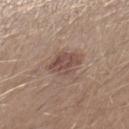notes=catalogued during a skin exam; not biopsied
patient=male, about 30 years old
acquisition=total-body-photography crop, ~15 mm field of view
lighting=white-light
location=the right lower leg